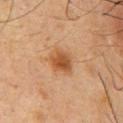Impression:
Part of a total-body skin-imaging series; this lesion was reviewed on a skin check and was not flagged for biopsy.
Background:
Imaged with cross-polarized lighting. Measured at roughly 3 mm in maximum diameter. An algorithmic analysis of the crop reported a lesion area of about 7.5 mm², an eccentricity of roughly 0.5, and two-axis asymmetry of about 0.2. The analysis additionally found an automated nevus-likeness rating near 90 out of 100 and lesion-presence confidence of about 100/100. On the chest. A male subject aged 58 to 62. A 15 mm crop from a total-body photograph taken for skin-cancer surveillance.